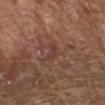Captured during whole-body skin photography for melanoma surveillance; the lesion was not biopsied.
Cropped from a whole-body photographic skin survey; the tile spans about 15 mm.
The subject is a male aged 63–67.
The tile uses cross-polarized illumination.
Located on the right forearm.
Longest diameter approximately 3 mm.